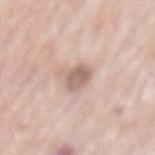Imaged during a routine full-body skin examination; the lesion was not biopsied and no histopathology is available.
The subject is a male aged 78 to 82.
Approximately 3 mm at its widest.
From the back.
A 15 mm crop from a total-body photograph taken for skin-cancer surveillance.
Imaged with white-light lighting.